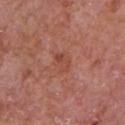Q: Was this lesion biopsied?
A: total-body-photography surveillance lesion; no biopsy
Q: Patient demographics?
A: male, in their mid- to late 60s
Q: What is the anatomic site?
A: the chest
Q: What lighting was used for the tile?
A: white-light
Q: What did automated image analysis measure?
A: a within-lesion color-variation index near 0/10
Q: What kind of image is this?
A: total-body-photography crop, ~15 mm field of view
Q: What is the lesion's diameter?
A: ~2.5 mm (longest diameter)